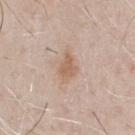Assessment: Imaged during a routine full-body skin examination; the lesion was not biopsied and no histopathology is available. Background: A region of skin cropped from a whole-body photographic capture, roughly 15 mm wide. The lesion is located on the chest. A male subject aged 63–67. An algorithmic analysis of the crop reported a nevus-likeness score of about 50/100 and a detector confidence of about 100 out of 100 that the crop contains a lesion. The tile uses white-light illumination. About 3.5 mm across.